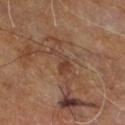The lesion was photographed on a routine skin check and not biopsied; there is no pathology result. The patient is a male about 60 years old. Cropped from a total-body skin-imaging series; the visible field is about 15 mm. From the right leg.